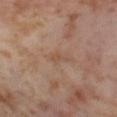Clinical impression: Recorded during total-body skin imaging; not selected for excision or biopsy. Acquisition and patient details: The lesion is on the right thigh. A 15 mm crop from a total-body photograph taken for skin-cancer surveillance. The patient is a female about 55 years old.